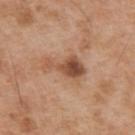The lesion was tiled from a total-body skin photograph and was not biopsied. The lesion is on the upper back. A male patient, in their mid- to late 50s. A close-up tile cropped from a whole-body skin photograph, about 15 mm across.